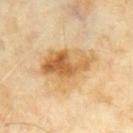Recorded during total-body skin imaging; not selected for excision or biopsy.
On the mid back.
This is a cross-polarized tile.
A male subject, about 60 years old.
A region of skin cropped from a whole-body photographic capture, roughly 15 mm wide.
Measured at roughly 7 mm in maximum diameter.